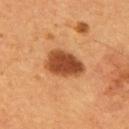Part of a total-body skin-imaging series; this lesion was reviewed on a skin check and was not flagged for biopsy. The lesion is on the upper back. About 5 mm across. Imaged with cross-polarized lighting. A close-up tile cropped from a whole-body skin photograph, about 15 mm across. Automated tile analysis of the lesion measured an area of roughly 13 mm² and two-axis asymmetry of about 0.2. And it measured a border-irregularity index near 2/10, a within-lesion color-variation index near 4/10, and a peripheral color-asymmetry measure near 1. It also reported a classifier nevus-likeness of about 100/100. A male subject, about 55 years old.